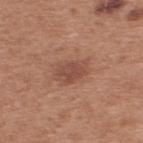Clinical impression:
This lesion was catalogued during total-body skin photography and was not selected for biopsy.
Acquisition and patient details:
The patient is a male aged around 55. This image is a 15 mm lesion crop taken from a total-body photograph. About 3 mm across. The lesion is located on the upper back. The tile uses white-light illumination.